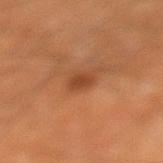– biopsy status — no biopsy performed (imaged during a skin exam)
– illumination — cross-polarized illumination
– patient — male, roughly 65 years of age
– automated metrics — an average lesion color of about L≈39 a*≈24 b*≈34 (CIELAB) and a lesion-to-skin contrast of about 7 (normalized; higher = more distinct); a detector confidence of about 100 out of 100 that the crop contains a lesion
– location — the left lower leg
– imaging modality — 15 mm crop, total-body photography